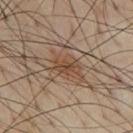notes: imaged on a skin check; not biopsied
acquisition: total-body-photography crop, ~15 mm field of view
anatomic site: the front of the torso
automated metrics: a footprint of about 4 mm², an outline eccentricity of about 0.9 (0 = round, 1 = elongated), and two-axis asymmetry of about 0.4; an average lesion color of about L≈45 a*≈17 b*≈30 (CIELAB), about 8 CIELAB-L* units darker than the surrounding skin, and a normalized border contrast of about 7.5; a border-irregularity rating of about 4/10, a within-lesion color-variation index near 1/10, and a peripheral color-asymmetry measure near 0.5; a classifier nevus-likeness of about 60/100 and lesion-presence confidence of about 90/100
subject: male, aged 53–57
lighting: cross-polarized illumination
lesion size: ~3 mm (longest diameter)